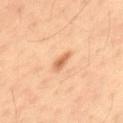Clinical impression: The lesion was photographed on a routine skin check and not biopsied; there is no pathology result. Background: Cropped from a whole-body photographic skin survey; the tile spans about 15 mm. A male subject, aged 33–37. The lesion is located on the lower back. Approximately 3 mm at its widest. Imaged with cross-polarized lighting.